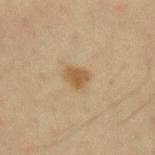• workup: no biopsy performed (imaged during a skin exam)
• subject: male, aged 58–62
• TBP lesion metrics: a lesion area of about 4 mm², a shape eccentricity near 0.6, and a symmetry-axis asymmetry near 0.3; a border-irregularity rating of about 3/10 and internal color variation of about 2 on a 0–10 scale; a nevus-likeness score of about 80/100
• image: 15 mm crop, total-body photography
• location: the left forearm
• lesion size: ~2.5 mm (longest diameter)